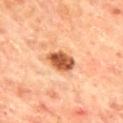Recorded during total-body skin imaging; not selected for excision or biopsy. A male subject aged 63–67. Longest diameter approximately 3.5 mm. The tile uses cross-polarized illumination. On the mid back. A roughly 15 mm field-of-view crop from a total-body skin photograph. An algorithmic analysis of the crop reported a shape eccentricity near 0.7 and two-axis asymmetry of about 0.15. And it measured a nevus-likeness score of about 95/100 and a detector confidence of about 100 out of 100 that the crop contains a lesion.